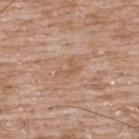workup: no biopsy performed (imaged during a skin exam) | site: the upper back | patient: male, aged around 50 | lesion diameter: ≈3 mm | imaging modality: 15 mm crop, total-body photography.